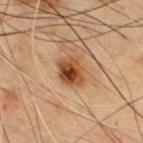Part of a total-body skin-imaging series; this lesion was reviewed on a skin check and was not flagged for biopsy.
Located on the chest.
The subject is a male roughly 55 years of age.
Captured under cross-polarized illumination.
The lesion-visualizer software estimated an area of roughly 8.5 mm², an eccentricity of roughly 0.6, and a symmetry-axis asymmetry near 0.15. The software also gave a mean CIELAB color near L≈39 a*≈19 b*≈31.
A 15 mm close-up tile from a total-body photography series done for melanoma screening.
Measured at roughly 3.5 mm in maximum diameter.